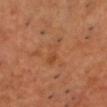notes: no biopsy performed (imaged during a skin exam)
automated lesion analysis: a border-irregularity index near 5/10 and peripheral color asymmetry of about 0.5; a nevus-likeness score of about 0/100 and lesion-presence confidence of about 100/100
size: ≈4 mm
lighting: cross-polarized
acquisition: ~15 mm crop, total-body skin-cancer survey
subject: male, about 50 years old
location: the chest